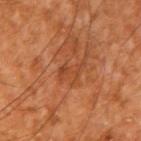workup: no biopsy performed (imaged during a skin exam)
image source: ~15 mm tile from a whole-body skin photo
illumination: cross-polarized illumination
site: the right upper arm
patient: aged 63–67